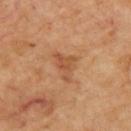biopsy status: no biopsy performed (imaged during a skin exam)
automated metrics: a lesion color around L≈51 a*≈23 b*≈36 in CIELAB, a lesion–skin lightness drop of about 8, and a normalized lesion–skin contrast near 6; internal color variation of about 4 on a 0–10 scale and peripheral color asymmetry of about 1.5
location: the upper back
tile lighting: cross-polarized illumination
image: 15 mm crop, total-body photography
patient: aged 63–67
lesion size: about 3.5 mm